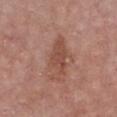Clinical impression:
The lesion was photographed on a routine skin check and not biopsied; there is no pathology result.
Image and clinical context:
Measured at roughly 6 mm in maximum diameter. A male subject aged 83 or over. The lesion is on the chest. A lesion tile, about 15 mm wide, cut from a 3D total-body photograph. Captured under white-light illumination.